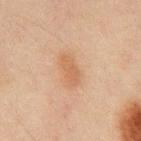<lesion>
  <biopsy_status>not biopsied; imaged during a skin examination</biopsy_status>
  <automated_metrics>
    <area_mm2_approx>6.0</area_mm2_approx>
    <eccentricity>0.9</eccentricity>
    <shape_asymmetry>0.25</shape_asymmetry>
    <cielab_L>49</cielab_L>
    <cielab_a>18</cielab_a>
    <cielab_b>30</cielab_b>
    <vs_skin_contrast_norm>6.0</vs_skin_contrast_norm>
    <border_irregularity_0_10>3.0</border_irregularity_0_10>
    <nevus_likeness_0_100>50</nevus_likeness_0_100>
    <lesion_detection_confidence_0_100>100</lesion_detection_confidence_0_100>
  </automated_metrics>
  <image>
    <source>total-body photography crop</source>
    <field_of_view_mm>15</field_of_view_mm>
  </image>
  <patient>
    <sex>male</sex>
    <age_approx>45</age_approx>
  </patient>
  <site>abdomen</site>
  <lesion_size>
    <long_diameter_mm_approx>4.0</long_diameter_mm_approx>
  </lesion_size>
</lesion>